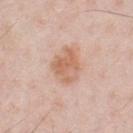The lesion was photographed on a routine skin check and not biopsied; there is no pathology result. A 15 mm crop from a total-body photograph taken for skin-cancer surveillance. Imaged with white-light lighting. The patient is a male in their 60s. The lesion is on the front of the torso.